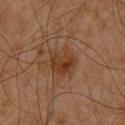Assessment: Imaged during a routine full-body skin examination; the lesion was not biopsied and no histopathology is available. Clinical summary: A male subject, approximately 60 years of age. A lesion tile, about 15 mm wide, cut from a 3D total-body photograph. The lesion is on the front of the torso. Longest diameter approximately 4 mm. The tile uses cross-polarized illumination.